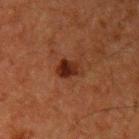The lesion is located on the left upper arm. The subject is a male aged around 60. Approximately 3 mm at its widest. Captured under cross-polarized illumination. A 15 mm close-up tile from a total-body photography series done for melanoma screening.